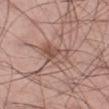Assessment: Captured during whole-body skin photography for melanoma surveillance; the lesion was not biopsied. Context: The recorded lesion diameter is about 4.5 mm. Automated tile analysis of the lesion measured a mean CIELAB color near L≈53 a*≈19 b*≈25, roughly 8 lightness units darker than nearby skin, and a normalized border contrast of about 6. And it measured a border-irregularity rating of about 3/10, internal color variation of about 6.5 on a 0–10 scale, and a peripheral color-asymmetry measure near 2. The analysis additionally found a classifier nevus-likeness of about 0/100. A 15 mm crop from a total-body photograph taken for skin-cancer surveillance. A male patient in their mid- to late 40s. Located on the right thigh. This is a white-light tile.